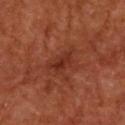Q: Was this lesion biopsied?
A: no biopsy performed (imaged during a skin exam)
Q: How was the tile lit?
A: cross-polarized
Q: What did automated image analysis measure?
A: an outline eccentricity of about 0.85 (0 = round, 1 = elongated) and a symmetry-axis asymmetry near 0.4
Q: Patient demographics?
A: male, aged 63 to 67
Q: Lesion location?
A: the back
Q: What is the imaging modality?
A: 15 mm crop, total-body photography